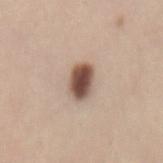Clinical summary:
On the mid back. The total-body-photography lesion software estimated a within-lesion color-variation index near 5/10 and radial color variation of about 1.5. A female patient aged around 40. A 15 mm crop from a total-body photograph taken for skin-cancer surveillance. Imaged with white-light lighting. Longest diameter approximately 4 mm.